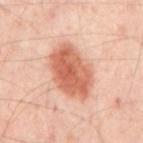workup = no biopsy performed (imaged during a skin exam)
patient = aged 53–57
image = 15 mm crop, total-body photography
tile lighting = cross-polarized
anatomic site = the mid back
lesion size = ~6.5 mm (longest diameter)